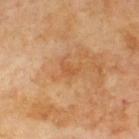Assessment: Imaged during a routine full-body skin examination; the lesion was not biopsied and no histopathology is available. Background: Located on the upper back. Cropped from a whole-body photographic skin survey; the tile spans about 15 mm. The patient is a male about 70 years old.